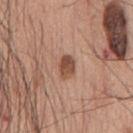Clinical impression:
This lesion was catalogued during total-body skin photography and was not selected for biopsy.
Acquisition and patient details:
Approximately 3 mm at its widest. A male patient, aged approximately 60. The lesion is on the chest. A roughly 15 mm field-of-view crop from a total-body skin photograph. The lesion-visualizer software estimated an area of roughly 5 mm². The analysis additionally found an average lesion color of about L≈50 a*≈22 b*≈29 (CIELAB) and about 12 CIELAB-L* units darker than the surrounding skin.